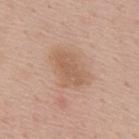Impression: No biopsy was performed on this lesion — it was imaged during a full skin examination and was not determined to be concerning. Background: This is a white-light tile. The subject is a male approximately 55 years of age. Cropped from a total-body skin-imaging series; the visible field is about 15 mm. The total-body-photography lesion software estimated an area of roughly 10 mm², an eccentricity of roughly 0.8, and a shape-asymmetry score of about 0.3 (0 = symmetric). The analysis additionally found a nevus-likeness score of about 10/100 and a lesion-detection confidence of about 100/100. The lesion is located on the mid back. Approximately 4.5 mm at its widest.